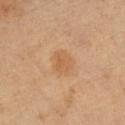Notes:
– follow-up · catalogued during a skin exam; not biopsied
– subject · female, in their 70s
– tile lighting · cross-polarized illumination
– site · the right lower leg
– image · ~15 mm crop, total-body skin-cancer survey
– lesion diameter · ≈2.5 mm